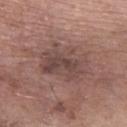Q: Was this lesion biopsied?
A: imaged on a skin check; not biopsied
Q: Lesion size?
A: about 6 mm
Q: How was this image acquired?
A: ~15 mm crop, total-body skin-cancer survey
Q: Where on the body is the lesion?
A: the right forearm
Q: What lighting was used for the tile?
A: white-light
Q: Who is the patient?
A: male, aged 58 to 62